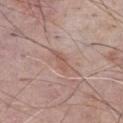Findings:
* imaging modality — ~15 mm crop, total-body skin-cancer survey
* location — the abdomen
* patient — male, aged approximately 75